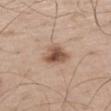{
  "biopsy_status": "not biopsied; imaged during a skin examination",
  "lighting": "white-light",
  "patient": {
    "sex": "male",
    "age_approx": 60
  },
  "site": "left thigh",
  "automated_metrics": {
    "area_mm2_approx": 7.5,
    "eccentricity": 0.65,
    "shape_asymmetry": 0.25,
    "color_variation_0_10": 5.5,
    "peripheral_color_asymmetry": 2.0,
    "nevus_likeness_0_100": 90,
    "lesion_detection_confidence_0_100": 100
  },
  "lesion_size": {
    "long_diameter_mm_approx": 3.5
  },
  "image": {
    "source": "total-body photography crop",
    "field_of_view_mm": 15
  }
}